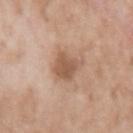biopsy status: total-body-photography surveillance lesion; no biopsy | imaging modality: ~15 mm tile from a whole-body skin photo | body site: the left upper arm | automated metrics: an area of roughly 8 mm², an outline eccentricity of about 0.7 (0 = round, 1 = elongated), and two-axis asymmetry of about 0.25; border irregularity of about 3 on a 0–10 scale and a color-variation rating of about 2/10; a nevus-likeness score of about 5/100 and lesion-presence confidence of about 100/100 | tile lighting: white-light | patient: male, approximately 50 years of age.